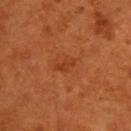Q: Was a biopsy performed?
A: total-body-photography surveillance lesion; no biopsy
Q: Lesion location?
A: the upper back
Q: How was this image acquired?
A: total-body-photography crop, ~15 mm field of view
Q: Patient demographics?
A: male, roughly 60 years of age
Q: How large is the lesion?
A: about 3 mm